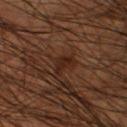Clinical impression: Part of a total-body skin-imaging series; this lesion was reviewed on a skin check and was not flagged for biopsy. Context: About 2.5 mm across. A male subject aged 43–47. A 15 mm close-up tile from a total-body photography series done for melanoma screening. Automated tile analysis of the lesion measured a mean CIELAB color near L≈22 a*≈18 b*≈23, about 6 CIELAB-L* units darker than the surrounding skin, and a lesion-to-skin contrast of about 7.5 (normalized; higher = more distinct). The software also gave a lesion-detection confidence of about 90/100. Located on the leg. This is a cross-polarized tile.